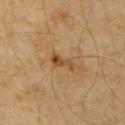Captured during whole-body skin photography for melanoma surveillance; the lesion was not biopsied. The total-body-photography lesion software estimated a lesion area of about 3.5 mm² and an eccentricity of roughly 0.9. The analysis additionally found a lesion-to-skin contrast of about 8 (normalized; higher = more distinct). And it measured a border-irregularity index near 5.5/10. And it measured an automated nevus-likeness rating near 30 out of 100 and a detector confidence of about 100 out of 100 that the crop contains a lesion. Cropped from a whole-body photographic skin survey; the tile spans about 15 mm. The tile uses cross-polarized illumination. Located on the arm. A male subject, about 60 years old.